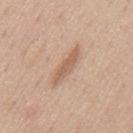Imaged during a routine full-body skin examination; the lesion was not biopsied and no histopathology is available.
An algorithmic analysis of the crop reported an area of roughly 5 mm², a shape eccentricity near 0.95, and a shape-asymmetry score of about 0.3 (0 = symmetric). And it measured a lesion–skin lightness drop of about 10.
The patient is a male in their 30s.
Measured at roughly 5 mm in maximum diameter.
The lesion is on the mid back.
A roughly 15 mm field-of-view crop from a total-body skin photograph.
Imaged with white-light lighting.